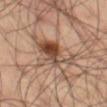This lesion was catalogued during total-body skin photography and was not selected for biopsy. About 5.5 mm across. Cropped from a whole-body photographic skin survey; the tile spans about 15 mm. The subject is a male aged 58 to 62. Captured under cross-polarized illumination. On the left thigh.